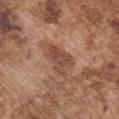biopsy status — total-body-photography surveillance lesion; no biopsy
image source — 15 mm crop, total-body photography
automated metrics — a mean CIELAB color near L≈47 a*≈21 b*≈29, roughly 10 lightness units darker than nearby skin, and a lesion-to-skin contrast of about 7.5 (normalized; higher = more distinct); a classifier nevus-likeness of about 0/100 and a detector confidence of about 100 out of 100 that the crop contains a lesion
body site — the left upper arm
size — about 4.5 mm
tile lighting — white-light illumination
patient — male, in their mid-70s